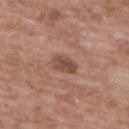Q: Lesion location?
A: the chest
Q: What is the imaging modality?
A: total-body-photography crop, ~15 mm field of view
Q: How was the tile lit?
A: white-light illumination
Q: Patient demographics?
A: male, roughly 60 years of age
Q: Lesion size?
A: ≈3.5 mm